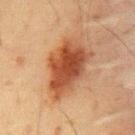follow-up — total-body-photography surveillance lesion; no biopsy
illumination — cross-polarized
location — the chest
lesion size — ≈7 mm
imaging modality — total-body-photography crop, ~15 mm field of view
patient — male, aged 58 to 62
TBP lesion metrics — a mean CIELAB color near L≈38 a*≈22 b*≈30, a lesion–skin lightness drop of about 12, and a lesion-to-skin contrast of about 10.5 (normalized; higher = more distinct); border irregularity of about 3.5 on a 0–10 scale and radial color variation of about 1.5; a nevus-likeness score of about 100/100 and lesion-presence confidence of about 100/100